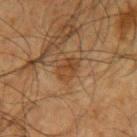Findings:
- subject — male, in their mid- to late 60s
- image — 15 mm crop, total-body photography
- anatomic site — the arm